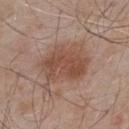Clinical impression:
The lesion was photographed on a routine skin check and not biopsied; there is no pathology result.
Acquisition and patient details:
The tile uses white-light illumination. On the upper back. The lesion's longest dimension is about 6.5 mm. The lesion-visualizer software estimated a footprint of about 25 mm², an outline eccentricity of about 0.7 (0 = round, 1 = elongated), and a shape-asymmetry score of about 0.2 (0 = symmetric). The analysis additionally found a border-irregularity index near 3/10 and radial color variation of about 1.5. A male subject aged 53 to 57. Cropped from a whole-body photographic skin survey; the tile spans about 15 mm.